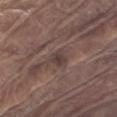The lesion was tiled from a total-body skin photograph and was not biopsied. A 15 mm crop from a total-body photograph taken for skin-cancer surveillance. Measured at roughly 3 mm in maximum diameter. The subject is a male roughly 80 years of age. The lesion is located on the arm.